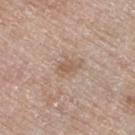biopsy status: no biopsy performed (imaged during a skin exam); image source: ~15 mm crop, total-body skin-cancer survey; patient: male, aged around 80; location: the right thigh.